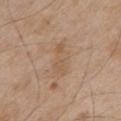Q: Who is the patient?
A: male, aged 63–67
Q: Illumination type?
A: white-light
Q: What kind of image is this?
A: ~15 mm crop, total-body skin-cancer survey
Q: What is the anatomic site?
A: the chest